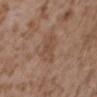Assessment: Captured during whole-body skin photography for melanoma surveillance; the lesion was not biopsied. Background: Approximately 4.5 mm at its widest. A female patient, in their mid- to late 30s. Located on the upper back. Cropped from a whole-body photographic skin survey; the tile spans about 15 mm.